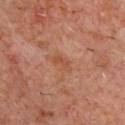<lesion>
  <biopsy_status>not biopsied; imaged during a skin examination</biopsy_status>
  <site>chest</site>
  <image>
    <source>total-body photography crop</source>
    <field_of_view_mm>15</field_of_view_mm>
  </image>
  <automated_metrics>
    <area_mm2_approx>3.0</area_mm2_approx>
    <eccentricity>0.9</eccentricity>
    <cielab_L>46</cielab_L>
    <cielab_a>23</cielab_a>
    <cielab_b>31</cielab_b>
    <vs_skin_darker_L>6.0</vs_skin_darker_L>
    <vs_skin_contrast_norm>5.5</vs_skin_contrast_norm>
    <border_irregularity_0_10>4.5</border_irregularity_0_10>
    <color_variation_0_10>0.0</color_variation_0_10>
    <peripheral_color_asymmetry>0.0</peripheral_color_asymmetry>
    <nevus_likeness_0_100>0</nevus_likeness_0_100>
    <lesion_detection_confidence_0_100>100</lesion_detection_confidence_0_100>
  </automated_metrics>
  <patient>
    <sex>male</sex>
    <age_approx>60</age_approx>
  </patient>
</lesion>